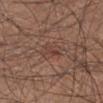Captured during whole-body skin photography for melanoma surveillance; the lesion was not biopsied. Captured under white-light illumination. The lesion's longest dimension is about 3 mm. On the right lower leg. A close-up tile cropped from a whole-body skin photograph, about 15 mm across. Automated tile analysis of the lesion measured an eccentricity of roughly 0.85 and a symmetry-axis asymmetry near 0.4. The software also gave about 6 CIELAB-L* units darker than the surrounding skin and a lesion-to-skin contrast of about 5 (normalized; higher = more distinct). It also reported an automated nevus-likeness rating near 0 out of 100. A male subject about 50 years old.